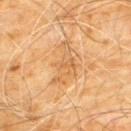- biopsy status · total-body-photography surveillance lesion; no biopsy
- tile lighting · cross-polarized
- patient · male, roughly 60 years of age
- site · the chest
- TBP lesion metrics · roughly 7 lightness units darker than nearby skin and a normalized border contrast of about 5; border irregularity of about 6 on a 0–10 scale and radial color variation of about 1; a nevus-likeness score of about 0/100
- acquisition · ~15 mm crop, total-body skin-cancer survey